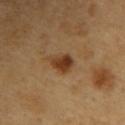Clinical impression: This lesion was catalogued during total-body skin photography and was not selected for biopsy. Context: Captured under cross-polarized illumination. Approximately 3.5 mm at its widest. Located on the arm. An algorithmic analysis of the crop reported a footprint of about 5.5 mm² and a symmetry-axis asymmetry near 0.35. The analysis additionally found a classifier nevus-likeness of about 95/100. A male patient aged 58 to 62. A 15 mm close-up tile from a total-body photography series done for melanoma screening.